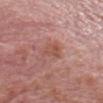On the head or neck. Imaged with white-light lighting. A roughly 15 mm field-of-view crop from a total-body skin photograph. A male patient aged 63 to 67. Automated tile analysis of the lesion measured a lesion area of about 4.5 mm² and a shape-asymmetry score of about 0.3 (0 = symmetric). The analysis additionally found a nevus-likeness score of about 0/100 and lesion-presence confidence of about 100/100. The lesion's longest dimension is about 3.5 mm.